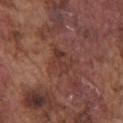The lesion was photographed on a routine skin check and not biopsied; there is no pathology result. This image is a 15 mm lesion crop taken from a total-body photograph. A male subject about 75 years old. Imaged with white-light lighting. Located on the chest. About 3.5 mm across. An algorithmic analysis of the crop reported a border-irregularity index near 3.5/10, internal color variation of about 4 on a 0–10 scale, and peripheral color asymmetry of about 1.5. It also reported a nevus-likeness score of about 0/100 and lesion-presence confidence of about 95/100.